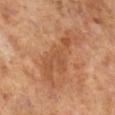This lesion was catalogued during total-body skin photography and was not selected for biopsy.